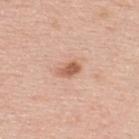Imaged during a routine full-body skin examination; the lesion was not biopsied and no histopathology is available.
The lesion is on the upper back.
The recorded lesion diameter is about 3 mm.
Cropped from a whole-body photographic skin survey; the tile spans about 15 mm.
Captured under white-light illumination.
A male subject approximately 35 years of age.
Automated tile analysis of the lesion measured a footprint of about 3.5 mm², a shape eccentricity near 0.8, and a shape-asymmetry score of about 0.3 (0 = symmetric). And it measured a border-irregularity rating of about 3/10, internal color variation of about 3 on a 0–10 scale, and peripheral color asymmetry of about 1.